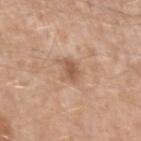notes: imaged on a skin check; not biopsied | patient: male, aged 53–57 | imaging modality: total-body-photography crop, ~15 mm field of view | lesion size: about 3 mm | tile lighting: white-light illumination | automated lesion analysis: a lesion area of about 4.5 mm²; a lesion color around L≈56 a*≈20 b*≈31 in CIELAB, a lesion–skin lightness drop of about 10, and a normalized lesion–skin contrast near 6.5; border irregularity of about 3 on a 0–10 scale, a within-lesion color-variation index near 1.5/10, and peripheral color asymmetry of about 0.5 | body site: the left upper arm.